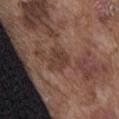Notes:
• notes: no biopsy performed (imaged during a skin exam)
• tile lighting: white-light illumination
• acquisition: total-body-photography crop, ~15 mm field of view
• image-analysis metrics: an average lesion color of about L≈38 a*≈18 b*≈23 (CIELAB) and a lesion–skin lightness drop of about 8; a border-irregularity index near 3/10 and peripheral color asymmetry of about 1
• size: ≈2.5 mm
• body site: the abdomen
• subject: male, roughly 75 years of age